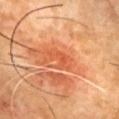Impression: The lesion was photographed on a routine skin check and not biopsied; there is no pathology result. Background: A male patient aged around 60. The lesion's longest dimension is about 3.5 mm. Automated tile analysis of the lesion measured an average lesion color of about L≈53 a*≈32 b*≈40 (CIELAB) and about 7 CIELAB-L* units darker than the surrounding skin. The analysis additionally found a color-variation rating of about 2/10 and a peripheral color-asymmetry measure near 0.5. The analysis additionally found an automated nevus-likeness rating near 0 out of 100 and a lesion-detection confidence of about 95/100. Captured under cross-polarized illumination. A lesion tile, about 15 mm wide, cut from a 3D total-body photograph. The lesion is located on the chest.